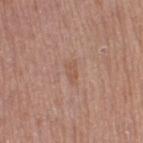Q: Is there a histopathology result?
A: imaged on a skin check; not biopsied